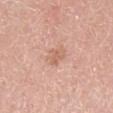Captured during whole-body skin photography for melanoma surveillance; the lesion was not biopsied.
The lesion is located on the leg.
A male subject aged approximately 70.
A region of skin cropped from a whole-body photographic capture, roughly 15 mm wide.
This is a white-light tile.